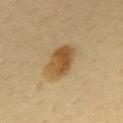A 15 mm crop from a total-body photograph taken for skin-cancer surveillance. Automated image analysis of the tile measured an area of roughly 11 mm², an outline eccentricity of about 0.8 (0 = round, 1 = elongated), and a symmetry-axis asymmetry near 0.15. It also reported a lesion color around L≈50 a*≈16 b*≈38 in CIELAB and a normalized lesion–skin contrast near 9. The software also gave a border-irregularity rating of about 1.5/10, a within-lesion color-variation index near 5.5/10, and peripheral color asymmetry of about 2. The analysis additionally found a classifier nevus-likeness of about 100/100 and lesion-presence confidence of about 100/100. The lesion is located on the mid back. About 4.5 mm across. A female patient approximately 50 years of age.